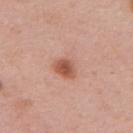subject: female, in their 50s; image source: ~15 mm tile from a whole-body skin photo; location: the upper back; lesion size: ~4 mm (longest diameter); lighting: white-light illumination.